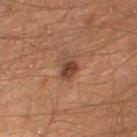biopsy status — total-body-photography surveillance lesion; no biopsy | site — the right thigh | lesion size — ~2.5 mm (longest diameter) | patient — male, in their mid- to late 40s | lighting — cross-polarized | image source — ~15 mm crop, total-body skin-cancer survey.